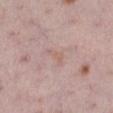biopsy status — imaged on a skin check; not biopsied.